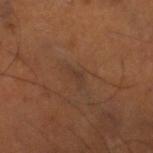Captured during whole-body skin photography for melanoma surveillance; the lesion was not biopsied. A male patient approximately 50 years of age. A 15 mm crop from a total-body photograph taken for skin-cancer surveillance. Longest diameter approximately 3 mm. An algorithmic analysis of the crop reported an average lesion color of about L≈35 a*≈17 b*≈26 (CIELAB), about 5 CIELAB-L* units darker than the surrounding skin, and a lesion-to-skin contrast of about 5 (normalized; higher = more distinct). It also reported a border-irregularity rating of about 5/10, a within-lesion color-variation index near 0/10, and a peripheral color-asymmetry measure near 0. The analysis additionally found an automated nevus-likeness rating near 0 out of 100 and a detector confidence of about 80 out of 100 that the crop contains a lesion. The lesion is located on the right lower leg. This is a cross-polarized tile.